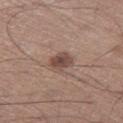biopsy_status: not biopsied; imaged during a skin examination
image:
  source: total-body photography crop
  field_of_view_mm: 15
automated_metrics:
  area_mm2_approx: 5.0
  eccentricity: 0.5
  shape_asymmetry: 0.2
lighting: white-light
site: left thigh
lesion_size:
  long_diameter_mm_approx: 2.5
patient:
  sex: male
  age_approx: 65